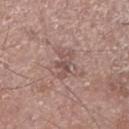Case summary:
* biopsy status — total-body-photography surveillance lesion; no biopsy
* automated metrics — a lesion color around L≈51 a*≈18 b*≈22 in CIELAB, roughly 8 lightness units darker than nearby skin, and a normalized border contrast of about 6; border irregularity of about 6 on a 0–10 scale, internal color variation of about 1.5 on a 0–10 scale, and a peripheral color-asymmetry measure near 0; a lesion-detection confidence of about 90/100
* anatomic site — the right lower leg
* image — total-body-photography crop, ~15 mm field of view
* subject — male, aged around 70
* lighting — white-light illumination